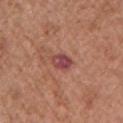No biopsy was performed on this lesion — it was imaged during a full skin examination and was not determined to be concerning. About 3 mm across. Imaged with white-light lighting. A female subject, about 75 years old. An algorithmic analysis of the crop reported a lesion area of about 4.5 mm², a shape eccentricity near 0.75, and a symmetry-axis asymmetry near 0.2. It also reported a border-irregularity index near 2/10, internal color variation of about 4 on a 0–10 scale, and radial color variation of about 1.5. From the left upper arm. This image is a 15 mm lesion crop taken from a total-body photograph.